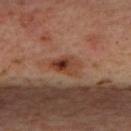<tbp_lesion>
  <biopsy_status>not biopsied; imaged during a skin examination</biopsy_status>
  <patient>
    <sex>female</sex>
    <age_approx>45</age_approx>
  </patient>
  <image>
    <source>total-body photography crop</source>
    <field_of_view_mm>15</field_of_view_mm>
  </image>
  <site>upper back</site>
  <lesion_size>
    <long_diameter_mm_approx>3.5</long_diameter_mm_approx>
  </lesion_size>
  <lighting>cross-polarized</lighting>
</tbp_lesion>